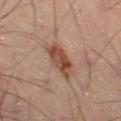workup=no biopsy performed (imaged during a skin exam); site=the leg; patient=male, aged 48–52; image source=~15 mm crop, total-body skin-cancer survey.